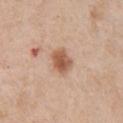<lesion>
<biopsy_status>not biopsied; imaged during a skin examination</biopsy_status>
<automated_metrics>
  <shape_asymmetry>0.2</shape_asymmetry>
  <border_irregularity_0_10>2.0</border_irregularity_0_10>
  <color_variation_0_10>4.0</color_variation_0_10>
</automated_metrics>
<patient>
  <sex>male</sex>
  <age_approx>60</age_approx>
</patient>
<lighting>white-light</lighting>
<site>chest</site>
<image>
  <source>total-body photography crop</source>
  <field_of_view_mm>15</field_of_view_mm>
</image>
<lesion_size>
  <long_diameter_mm_approx>3.0</long_diameter_mm_approx>
</lesion_size>
</lesion>